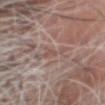| key | value |
|---|---|
| notes | imaged on a skin check; not biopsied |
| lesion diameter | ~2 mm (longest diameter) |
| subject | male, in their mid- to late 60s |
| imaging modality | total-body-photography crop, ~15 mm field of view |
| body site | the head or neck |
| illumination | white-light illumination |